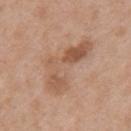{"site": "chest", "patient": {"sex": "male", "age_approx": 50}, "image": {"source": "total-body photography crop", "field_of_view_mm": 15}}